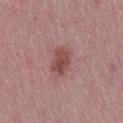A roughly 15 mm field-of-view crop from a total-body skin photograph.
Automated image analysis of the tile measured a border-irregularity index near 2/10 and a color-variation rating of about 3.5/10. The software also gave a classifier nevus-likeness of about 45/100.
The tile uses white-light illumination.
From the right thigh.
Measured at roughly 3.5 mm in maximum diameter.
A female patient, aged 43 to 47.